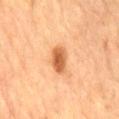biopsy status = no biopsy performed (imaged during a skin exam)
diameter = about 3 mm
imaging modality = ~15 mm tile from a whole-body skin photo
tile lighting = cross-polarized
patient = male, roughly 85 years of age or older
location = the mid back